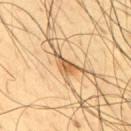The lesion was photographed on a routine skin check and not biopsied; there is no pathology result.
A roughly 15 mm field-of-view crop from a total-body skin photograph.
The lesion is on the back.
A male patient aged 43 to 47.